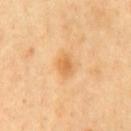| field | value |
|---|---|
| follow-up | imaged on a skin check; not biopsied |
| patient | male, aged 48 to 52 |
| anatomic site | the chest |
| lesion size | ≈2.5 mm |
| illumination | cross-polarized illumination |
| image source | 15 mm crop, total-body photography |